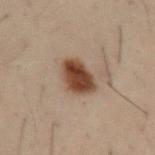Case summary:
* follow-up — catalogued during a skin exam; not biopsied
* lighting — cross-polarized illumination
* imaging modality — 15 mm crop, total-body photography
* lesion size — ~4 mm (longest diameter)
* location — the left upper arm
* patient — male, aged around 30
* image-analysis metrics — a footprint of about 10 mm²; an average lesion color of about L≈35 a*≈16 b*≈24 (CIELAB) and a normalized border contrast of about 12; border irregularity of about 2 on a 0–10 scale, a color-variation rating of about 4.5/10, and peripheral color asymmetry of about 1.5